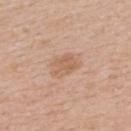Q: Is there a histopathology result?
A: total-body-photography surveillance lesion; no biopsy
Q: What is the lesion's diameter?
A: ≈3.5 mm
Q: What are the patient's age and sex?
A: female, aged 43 to 47
Q: What is the imaging modality?
A: total-body-photography crop, ~15 mm field of view
Q: Where on the body is the lesion?
A: the upper back
Q: Illumination type?
A: white-light